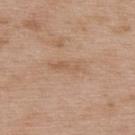{"biopsy_status": "not biopsied; imaged during a skin examination", "automated_metrics": {"area_mm2_approx": 4.5, "eccentricity": 0.95, "shape_asymmetry": 0.45, "cielab_L": 58, "cielab_a": 18, "cielab_b": 32, "vs_skin_darker_L": 6.0}, "lighting": "white-light", "patient": {"sex": "male", "age_approx": 50}, "site": "upper back", "lesion_size": {"long_diameter_mm_approx": 4.0}, "image": {"source": "total-body photography crop", "field_of_view_mm": 15}}